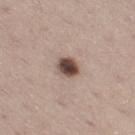Case summary:
• workup — total-body-photography surveillance lesion; no biopsy
• acquisition — 15 mm crop, total-body photography
• subject — male, aged 58 to 62
• anatomic site — the right thigh